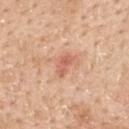No biopsy was performed on this lesion — it was imaged during a full skin examination and was not determined to be concerning. A region of skin cropped from a whole-body photographic capture, roughly 15 mm wide. The subject is a male aged 58–62. Approximately 3 mm at its widest. The lesion is on the upper back. An algorithmic analysis of the crop reported a footprint of about 4.5 mm², a shape eccentricity near 0.8, and a shape-asymmetry score of about 0.4 (0 = symmetric). It also reported a lesion color around L≈62 a*≈26 b*≈33 in CIELAB, about 10 CIELAB-L* units darker than the surrounding skin, and a normalized lesion–skin contrast near 6. It also reported a border-irregularity index near 3.5/10 and internal color variation of about 3 on a 0–10 scale. The software also gave a classifier nevus-likeness of about 20/100.